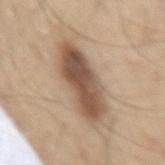follow-up — no biopsy performed (imaged during a skin exam)
subject — male, in their 60s
site — the right thigh
tile lighting — white-light illumination
image — ~15 mm tile from a whole-body skin photo
automated metrics — an area of roughly 21 mm², an eccentricity of roughly 0.95, and two-axis asymmetry of about 0.2; a border-irregularity rating of about 3/10, a within-lesion color-variation index near 6/10, and radial color variation of about 2; a nevus-likeness score of about 80/100 and a detector confidence of about 100 out of 100 that the crop contains a lesion
lesion size — about 9 mm